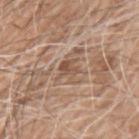Clinical impression: Part of a total-body skin-imaging series; this lesion was reviewed on a skin check and was not flagged for biopsy. Background: This image is a 15 mm lesion crop taken from a total-body photograph. Longest diameter approximately 3.5 mm. A male patient, in their 60s. Automated tile analysis of the lesion measured a lesion area of about 5 mm², a shape eccentricity near 0.65, and a symmetry-axis asymmetry near 0.35. And it measured an automated nevus-likeness rating near 0 out of 100 and a lesion-detection confidence of about 65/100. The lesion is on the left upper arm.